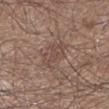  biopsy_status: not biopsied; imaged during a skin examination
  lesion_size:
    long_diameter_mm_approx: 4.5
  automated_metrics:
    cielab_L: 47
    cielab_a: 17
    cielab_b: 23
    vs_skin_darker_L: 6.0
    vs_skin_contrast_norm: 5.0
    lesion_detection_confidence_0_100: 85
  site: left lower leg
  image:
    source: total-body photography crop
    field_of_view_mm: 15
  patient:
    sex: male
    age_approx: 50
  lighting: white-light